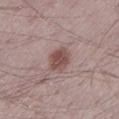Captured during whole-body skin photography for melanoma surveillance; the lesion was not biopsied. A 15 mm close-up extracted from a 3D total-body photography capture. Automated tile analysis of the lesion measured an area of roughly 7 mm² and an outline eccentricity of about 0.7 (0 = round, 1 = elongated). The analysis additionally found a peripheral color-asymmetry measure near 1. The tile uses white-light illumination. About 3.5 mm across. Located on the left lower leg. A male subject, aged approximately 50.